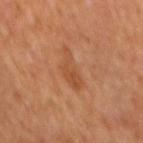This lesion was catalogued during total-body skin photography and was not selected for biopsy. About 5 mm across. Located on the back. The patient is a male about 65 years old. Captured under cross-polarized illumination. The total-body-photography lesion software estimated a lesion–skin lightness drop of about 7 and a normalized lesion–skin contrast near 5.5. It also reported a color-variation rating of about 2/10 and a peripheral color-asymmetry measure near 0.5. A lesion tile, about 15 mm wide, cut from a 3D total-body photograph.